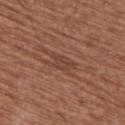Recorded during total-body skin imaging; not selected for excision or biopsy.